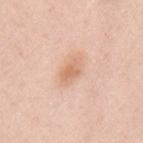The lesion was photographed on a routine skin check and not biopsied; there is no pathology result.
On the back.
A lesion tile, about 15 mm wide, cut from a 3D total-body photograph.
A female subject approximately 30 years of age.